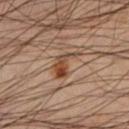<tbp_lesion>
<biopsy_status>not biopsied; imaged during a skin examination</biopsy_status>
<site>left lower leg</site>
<image>
  <source>total-body photography crop</source>
  <field_of_view_mm>15</field_of_view_mm>
</image>
<patient>
  <sex>male</sex>
  <age_approx>50</age_approx>
</patient>
<lighting>cross-polarized</lighting>
<automated_metrics>
  <area_mm2_approx>6.5</area_mm2_approx>
  <eccentricity>0.85</eccentricity>
  <nevus_likeness_0_100>90</nevus_likeness_0_100>
  <lesion_detection_confidence_0_100>100</lesion_detection_confidence_0_100>
</automated_metrics>
</tbp_lesion>